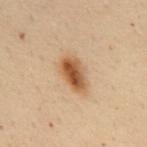Assessment:
The lesion was photographed on a routine skin check and not biopsied; there is no pathology result.
Clinical summary:
A female patient in their 50s. A 15 mm close-up tile from a total-body photography series done for melanoma screening. Captured under cross-polarized illumination. Located on the mid back. The lesion's longest dimension is about 4 mm.